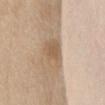<record>
  <lesion_size>
    <long_diameter_mm_approx>4.0</long_diameter_mm_approx>
  </lesion_size>
  <automated_metrics>
    <vs_skin_darker_L>10.0</vs_skin_darker_L>
    <vs_skin_contrast_norm>7.0</vs_skin_contrast_norm>
    <border_irregularity_0_10>2.0</border_irregularity_0_10>
    <color_variation_0_10>3.0</color_variation_0_10>
    <peripheral_color_asymmetry>1.0</peripheral_color_asymmetry>
    <nevus_likeness_0_100>0</nevus_likeness_0_100>
    <lesion_detection_confidence_0_100>100</lesion_detection_confidence_0_100>
  </automated_metrics>
  <patient>
    <sex>female</sex>
    <age_approx>65</age_approx>
  </patient>
  <image>
    <source>total-body photography crop</source>
    <field_of_view_mm>15</field_of_view_mm>
  </image>
  <lighting>white-light</lighting>
  <site>chest</site>
</record>